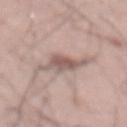| field | value |
|---|---|
| workup | imaged on a skin check; not biopsied |
| acquisition | 15 mm crop, total-body photography |
| site | the abdomen |
| subject | male, aged approximately 55 |
| tile lighting | white-light |
| lesion diameter | ≈6 mm |